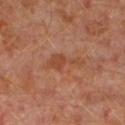Notes:
- follow-up · no biopsy performed (imaged during a skin exam)
- patient · male, aged approximately 30
- tile lighting · cross-polarized
- image source · total-body-photography crop, ~15 mm field of view
- location · the left lower leg
- TBP lesion metrics · an outline eccentricity of about 0.95 (0 = round, 1 = elongated) and a symmetry-axis asymmetry near 0.6; an average lesion color of about L≈45 a*≈25 b*≈32 (CIELAB), roughly 7 lightness units darker than nearby skin, and a normalized border contrast of about 6; a nevus-likeness score of about 0/100 and lesion-presence confidence of about 100/100